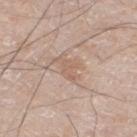Part of a total-body skin-imaging series; this lesion was reviewed on a skin check and was not flagged for biopsy.
The tile uses white-light illumination.
A male subject aged 58–62.
On the right thigh.
A roughly 15 mm field-of-view crop from a total-body skin photograph.
Longest diameter approximately 4 mm.